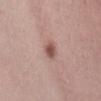follow-up = imaged on a skin check; not biopsied | anatomic site = the front of the torso | TBP lesion metrics = a border-irregularity rating of about 1.5/10 and a color-variation rating of about 2/10 | patient = female, aged around 60 | image source = ~15 mm crop, total-body skin-cancer survey | illumination = white-light illumination | lesion diameter = ~3 mm (longest diameter).